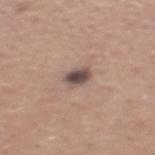| field | value |
|---|---|
| follow-up | total-body-photography surveillance lesion; no biopsy |
| lesion diameter | ≈2.5 mm |
| patient | male, aged 43–47 |
| tile lighting | white-light illumination |
| anatomic site | the mid back |
| acquisition | total-body-photography crop, ~15 mm field of view |